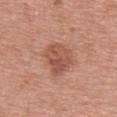Clinical impression:
The lesion was photographed on a routine skin check and not biopsied; there is no pathology result.
Context:
A roughly 15 mm field-of-view crop from a total-body skin photograph. Approximately 5 mm at its widest. The lesion is located on the upper back. The subject is a female aged 68–72.